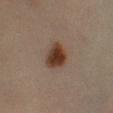notes: imaged on a skin check; not biopsied
patient: female, aged 68–72
image: 15 mm crop, total-body photography
tile lighting: cross-polarized illumination
diameter: ~3.5 mm (longest diameter)
automated lesion analysis: an average lesion color of about L≈32 a*≈16 b*≈24 (CIELAB), a lesion–skin lightness drop of about 12, and a lesion-to-skin contrast of about 12 (normalized; higher = more distinct)
body site: the left lower leg